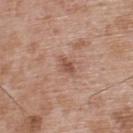Q: Was a biopsy performed?
A: imaged on a skin check; not biopsied
Q: What is the imaging modality?
A: total-body-photography crop, ~15 mm field of view
Q: Who is the patient?
A: male, roughly 50 years of age
Q: Lesion location?
A: the upper back
Q: Illumination type?
A: white-light
Q: Automated lesion metrics?
A: a border-irregularity index near 2/10 and peripheral color asymmetry of about 0.5; a nevus-likeness score of about 5/100
Q: Lesion size?
A: ~2.5 mm (longest diameter)